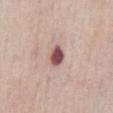No biopsy was performed on this lesion — it was imaged during a full skin examination and was not determined to be concerning. From the abdomen. A 15 mm close-up extracted from a 3D total-body photography capture. A male subject, roughly 75 years of age. The recorded lesion diameter is about 2.5 mm. Automated image analysis of the tile measured a lesion area of about 4.5 mm², an eccentricity of roughly 0.6, and two-axis asymmetry of about 0.15. The analysis additionally found a classifier nevus-likeness of about 0/100. Captured under white-light illumination.